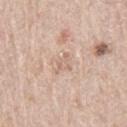Located on the right thigh. A roughly 15 mm field-of-view crop from a total-body skin photograph. The tile uses white-light illumination. Longest diameter approximately 3 mm. The lesion-visualizer software estimated a footprint of about 3.5 mm² and two-axis asymmetry of about 0.35. And it measured an average lesion color of about L≈66 a*≈17 b*≈28 (CIELAB) and a lesion-to-skin contrast of about 4.5 (normalized; higher = more distinct). The analysis additionally found a border-irregularity rating of about 4.5/10, a within-lesion color-variation index near 1.5/10, and a peripheral color-asymmetry measure near 0.5. The software also gave a classifier nevus-likeness of about 0/100 and lesion-presence confidence of about 100/100. The patient is a male approximately 80 years of age.